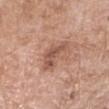Recorded during total-body skin imaging; not selected for excision or biopsy.
A female subject, approximately 75 years of age.
A region of skin cropped from a whole-body photographic capture, roughly 15 mm wide.
Automated image analysis of the tile measured about 11 CIELAB-L* units darker than the surrounding skin and a normalized lesion–skin contrast near 7.
The tile uses white-light illumination.
Located on the right forearm.
Approximately 4 mm at its widest.